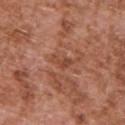{
  "automated_metrics": {
    "eccentricity": 0.9,
    "shape_asymmetry": 0.45,
    "vs_skin_darker_L": 8.0,
    "vs_skin_contrast_norm": 6.0,
    "border_irregularity_0_10": 5.0,
    "color_variation_0_10": 0.0,
    "peripheral_color_asymmetry": 0.0,
    "lesion_detection_confidence_0_100": 95
  },
  "site": "upper back",
  "image": {
    "source": "total-body photography crop",
    "field_of_view_mm": 15
  },
  "patient": {
    "sex": "male",
    "age_approx": 75
  },
  "lighting": "white-light"
}